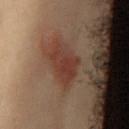Case summary:
– biopsy status — imaged on a skin check; not biopsied
– location — the abdomen
– patient — female, about 40 years old
– image — 15 mm crop, total-body photography
– automated metrics — an average lesion color of about L≈38 a*≈22 b*≈26 (CIELAB), a lesion–skin lightness drop of about 8, and a lesion-to-skin contrast of about 7 (normalized; higher = more distinct); a border-irregularity rating of about 3.5/10, internal color variation of about 4 on a 0–10 scale, and peripheral color asymmetry of about 1.5; lesion-presence confidence of about 100/100